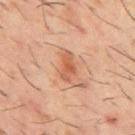workup: catalogued during a skin exam; not biopsied
site: the mid back
patient: male, aged 58–62
automated metrics: a footprint of about 5.5 mm² and two-axis asymmetry of about 0.3; border irregularity of about 3 on a 0–10 scale, internal color variation of about 4.5 on a 0–10 scale, and radial color variation of about 1; a nevus-likeness score of about 30/100 and a detector confidence of about 100 out of 100 that the crop contains a lesion
size: ≈4 mm
image: total-body-photography crop, ~15 mm field of view
lighting: cross-polarized